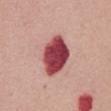{
  "biopsy_status": "not biopsied; imaged during a skin examination",
  "patient": {
    "sex": "female",
    "age_approx": 65
  },
  "site": "abdomen",
  "image": {
    "source": "total-body photography crop",
    "field_of_view_mm": 15
  },
  "lighting": "white-light",
  "automated_metrics": {
    "cielab_L": 45,
    "cielab_a": 36,
    "cielab_b": 21,
    "vs_skin_darker_L": 23.0,
    "vs_skin_contrast_norm": 15.0,
    "border_irregularity_0_10": 1.5,
    "peripheral_color_asymmetry": 1.5
  }
}